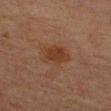<tbp_lesion>
  <biopsy_status>not biopsied; imaged during a skin examination</biopsy_status>
  <image>
    <source>total-body photography crop</source>
    <field_of_view_mm>15</field_of_view_mm>
  </image>
  <patient>
    <sex>male</sex>
    <age_approx>65</age_approx>
  </patient>
  <lighting>cross-polarized</lighting>
  <automated_metrics>
    <area_mm2_approx>8.5</area_mm2_approx>
    <eccentricity>0.7</eccentricity>
    <shape_asymmetry>0.2</shape_asymmetry>
    <cielab_L>30</cielab_L>
    <cielab_a>17</cielab_a>
    <cielab_b>26</cielab_b>
    <vs_skin_darker_L>6.0</vs_skin_darker_L>
    <vs_skin_contrast_norm>7.0</vs_skin_contrast_norm>
    <border_irregularity_0_10>2.0</border_irregularity_0_10>
    <color_variation_0_10>2.5</color_variation_0_10>
    <nevus_likeness_0_100>75</nevus_likeness_0_100>
    <lesion_detection_confidence_0_100>100</lesion_detection_confidence_0_100>
  </automated_metrics>
  <site>left upper arm</site>
</tbp_lesion>